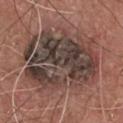The lesion's longest dimension is about 9 mm. The lesion-visualizer software estimated a border-irregularity rating of about 4/10, a within-lesion color-variation index near 9/10, and radial color variation of about 3.5. The lesion is located on the front of the torso. A male subject in their mid-70s. A lesion tile, about 15 mm wide, cut from a 3D total-body photograph. The tile uses white-light illumination.